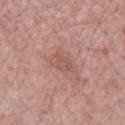biopsy status=total-body-photography surveillance lesion; no biopsy | lesion diameter=≈3 mm | patient=male, roughly 55 years of age | imaging modality=total-body-photography crop, ~15 mm field of view | tile lighting=white-light illumination.